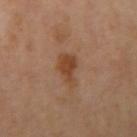{"biopsy_status": "not biopsied; imaged during a skin examination"}